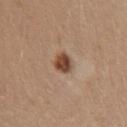Context:
Located on the abdomen. A 15 mm crop from a total-body photograph taken for skin-cancer surveillance. The tile uses white-light illumination. An algorithmic analysis of the crop reported a lesion area of about 5 mm². The lesion's longest dimension is about 3 mm. A female patient, roughly 45 years of age.